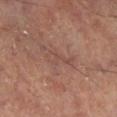The tile uses cross-polarized illumination. A close-up tile cropped from a whole-body skin photograph, about 15 mm across. The lesion is on the right lower leg. The lesion's longest dimension is about 4 mm.